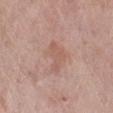Imaged during a routine full-body skin examination; the lesion was not biopsied and no histopathology is available.
A female patient aged approximately 70.
The lesion is located on the leg.
This image is a 15 mm lesion crop taken from a total-body photograph.
Automated image analysis of the tile measured a lesion area of about 6 mm² and an outline eccentricity of about 0.85 (0 = round, 1 = elongated). The analysis additionally found a mean CIELAB color near L≈57 a*≈21 b*≈26, a lesion–skin lightness drop of about 7, and a normalized border contrast of about 5. It also reported a border-irregularity index near 4.5/10, a color-variation rating of about 1.5/10, and radial color variation of about 0.5. The software also gave a classifier nevus-likeness of about 0/100.